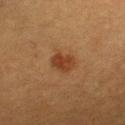subject = female, in their mid- to late 50s; diameter = ≈3.5 mm; imaging modality = 15 mm crop, total-body photography; tile lighting = cross-polarized; anatomic site = the chest.